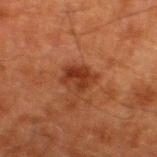biopsy status: no biopsy performed (imaged during a skin exam)
lesion diameter: ≈3.5 mm
body site: the right thigh
tile lighting: cross-polarized illumination
image: 15 mm crop, total-body photography
image-analysis metrics: an outline eccentricity of about 0.7 (0 = round, 1 = elongated) and a symmetry-axis asymmetry near 0.35; a mean CIELAB color near L≈29 a*≈23 b*≈29, roughly 8 lightness units darker than nearby skin, and a normalized border contrast of about 8.5; an automated nevus-likeness rating near 10 out of 100 and a detector confidence of about 100 out of 100 that the crop contains a lesion
subject: male, aged approximately 80